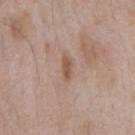biopsy_status: not biopsied; imaged during a skin examination
automated_metrics:
  vs_skin_darker_L: 10.0
  vs_skin_contrast_norm: 8.0
site: chest
image:
  source: total-body photography crop
  field_of_view_mm: 15
patient:
  sex: male
  age_approx: 65
lighting: white-light